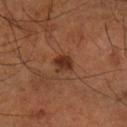Q: Was this lesion biopsied?
A: imaged on a skin check; not biopsied